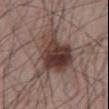Q: Is there a histopathology result?
A: no biopsy performed (imaged during a skin exam)
Q: What is the anatomic site?
A: the mid back
Q: Who is the patient?
A: male, about 65 years old
Q: What is the imaging modality?
A: 15 mm crop, total-body photography
Q: What is the lesion's diameter?
A: ~8 mm (longest diameter)